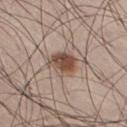The lesion was tiled from a total-body skin photograph and was not biopsied. This image is a 15 mm lesion crop taken from a total-body photograph. The lesion is located on the right thigh. This is a white-light tile. About 3.5 mm across. A male patient in their mid-30s.